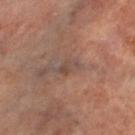  biopsy_status: not biopsied; imaged during a skin examination
  image:
    source: total-body photography crop
    field_of_view_mm: 15
  site: right leg
  patient:
    sex: female
    age_approx: 65
  lighting: cross-polarized